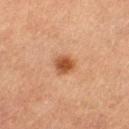Findings:
* notes · total-body-photography surveillance lesion; no biopsy
* image · ~15 mm crop, total-body skin-cancer survey
* diameter · ≈2.5 mm
* tile lighting · cross-polarized
* location · the left thigh
* subject · female, aged 58–62